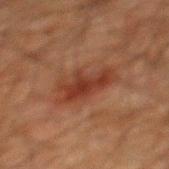lesion_size:
  long_diameter_mm_approx: 6.0
image:
  source: total-body photography crop
  field_of_view_mm: 15
lighting: cross-polarized
site: mid back
patient:
  sex: male
  age_approx: 60